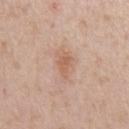biopsy status — total-body-photography surveillance lesion; no biopsy | image source — total-body-photography crop, ~15 mm field of view | body site — the chest | illumination — white-light | subject — male, in their mid- to late 50s | TBP lesion metrics — a mean CIELAB color near L≈60 a*≈20 b*≈32 and a lesion-to-skin contrast of about 6.5 (normalized; higher = more distinct) | lesion diameter — ~3.5 mm (longest diameter).